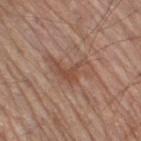Imaged during a routine full-body skin examination; the lesion was not biopsied and no histopathology is available. An algorithmic analysis of the crop reported a border-irregularity index near 7.5/10, internal color variation of about 4.5 on a 0–10 scale, and peripheral color asymmetry of about 1. The software also gave a classifier nevus-likeness of about 0/100 and a lesion-detection confidence of about 100/100. The subject is a male aged approximately 70. The lesion is on the right thigh. A 15 mm close-up tile from a total-body photography series done for melanoma screening. Measured at roughly 5.5 mm in maximum diameter.